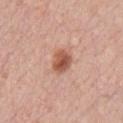Q: Was a biopsy performed?
A: total-body-photography surveillance lesion; no biopsy